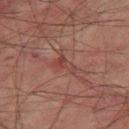– notes — imaged on a skin check; not biopsied
– image source — total-body-photography crop, ~15 mm field of view
– location — the right thigh
– automated metrics — a footprint of about 5 mm² and an outline eccentricity of about 0.85 (0 = round, 1 = elongated); a normalized lesion–skin contrast near 5.5
– illumination — cross-polarized illumination
– patient — male, aged 73–77
– lesion size — ≈3.5 mm